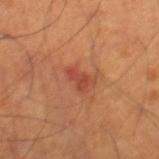Q: Was this lesion biopsied?
A: imaged on a skin check; not biopsied
Q: Automated lesion metrics?
A: border irregularity of about 5 on a 0–10 scale, internal color variation of about 1.5 on a 0–10 scale, and peripheral color asymmetry of about 0.5; an automated nevus-likeness rating near 20 out of 100
Q: How was the tile lit?
A: cross-polarized illumination
Q: Lesion location?
A: the right thigh
Q: What is the lesion's diameter?
A: ~3 mm (longest diameter)
Q: Patient demographics?
A: male, aged 68–72
Q: What kind of image is this?
A: total-body-photography crop, ~15 mm field of view